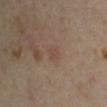This lesion was catalogued during total-body skin photography and was not selected for biopsy. A 15 mm close-up extracted from a 3D total-body photography capture. A male patient in their mid-40s. Located on the left upper arm.